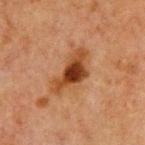Impression: Recorded during total-body skin imaging; not selected for excision or biopsy. Acquisition and patient details: A region of skin cropped from a whole-body photographic capture, roughly 15 mm wide. The lesion is located on the front of the torso. A male patient approximately 65 years of age. The tile uses cross-polarized illumination. Approximately 6 mm at its widest.